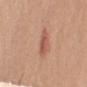follow-up: no biopsy performed (imaged during a skin exam)
acquisition: ~15 mm crop, total-body skin-cancer survey
subject: female, approximately 60 years of age
site: the right upper arm
automated metrics: roughly 9 lightness units darker than nearby skin and a lesion-to-skin contrast of about 6 (normalized; higher = more distinct); internal color variation of about 3 on a 0–10 scale and radial color variation of about 1